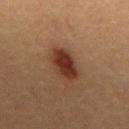{
  "biopsy_status": "not biopsied; imaged during a skin examination",
  "lighting": "cross-polarized",
  "automated_metrics": {
    "vs_skin_darker_L": 11.0
  },
  "site": "mid back",
  "image": {
    "source": "total-body photography crop",
    "field_of_view_mm": 15
  },
  "patient": {
    "sex": "male",
    "age_approx": 50
  }
}